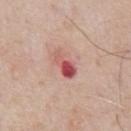workup: imaged on a skin check; not biopsied
subject: male, aged approximately 60
location: the front of the torso
imaging modality: ~15 mm crop, total-body skin-cancer survey
lesion diameter: ≈3.5 mm
lighting: white-light